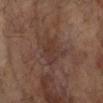biopsy status — catalogued during a skin exam; not biopsied